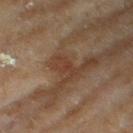- notes — imaged on a skin check; not biopsied
- anatomic site — the right thigh
- lesion size — ≈3 mm
- acquisition — 15 mm crop, total-body photography
- subject — female, roughly 60 years of age